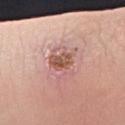| feature | finding |
|---|---|
| biopsy status | catalogued during a skin exam; not biopsied |
| body site | the arm |
| diameter | ~4.5 mm (longest diameter) |
| illumination | white-light illumination |
| imaging modality | ~15 mm tile from a whole-body skin photo |
| patient | male, in their mid- to late 20s |